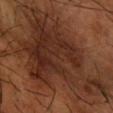| field | value |
|---|---|
| patient | male, approximately 65 years of age |
| site | the right forearm |
| image | ~15 mm tile from a whole-body skin photo |
| size | ~13.5 mm (longest diameter) |